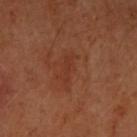Imaged during a routine full-body skin examination; the lesion was not biopsied and no histopathology is available. Measured at roughly 4 mm in maximum diameter. This is a cross-polarized tile. A 15 mm crop from a total-body photograph taken for skin-cancer surveillance. A female patient. An algorithmic analysis of the crop reported an average lesion color of about L≈37 a*≈26 b*≈32 (CIELAB), roughly 5 lightness units darker than nearby skin, and a lesion-to-skin contrast of about 5 (normalized; higher = more distinct). It also reported a border-irregularity rating of about 5.5/10. The software also gave a nevus-likeness score of about 0/100. The lesion is located on the left forearm.